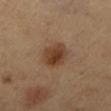biopsy_status: not biopsied; imaged during a skin examination
lesion_size:
  long_diameter_mm_approx: 4.0
lighting: cross-polarized
image:
  source: total-body photography crop
  field_of_view_mm: 15
site: left lower leg
patient:
  sex: female
  age_approx: 60